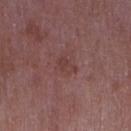notes: no biopsy performed (imaged during a skin exam) | subject: female, aged 68 to 72 | image: total-body-photography crop, ~15 mm field of view | location: the right upper arm | lesion diameter: about 2.5 mm | automated metrics: an automated nevus-likeness rating near 0 out of 100.